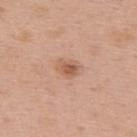Assessment: The lesion was tiled from a total-body skin photograph and was not biopsied. Context: Automated image analysis of the tile measured an automated nevus-likeness rating near 55 out of 100 and lesion-presence confidence of about 100/100. Located on the upper back. The patient is a female about 50 years old. A roughly 15 mm field-of-view crop from a total-body skin photograph. This is a white-light tile.